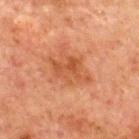{
  "lighting": "cross-polarized",
  "patient": {
    "sex": "male",
    "age_approx": 65
  },
  "image": {
    "source": "total-body photography crop",
    "field_of_view_mm": 15
  },
  "site": "chest",
  "lesion_size": {
    "long_diameter_mm_approx": 5.5
  },
  "automated_metrics": {
    "cielab_L": 43,
    "cielab_a": 24,
    "cielab_b": 33,
    "vs_skin_contrast_norm": 6.5,
    "border_irregularity_0_10": 4.0,
    "color_variation_0_10": 4.5,
    "peripheral_color_asymmetry": 1.5,
    "lesion_detection_confidence_0_100": 100
  }
}